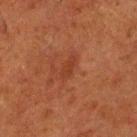Located on the left lower leg. A male subject aged 78 to 82. Automated image analysis of the tile measured a footprint of about 3.5 mm² and an eccentricity of roughly 0.9. Imaged with cross-polarized lighting. A lesion tile, about 15 mm wide, cut from a 3D total-body photograph.